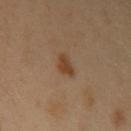Captured during whole-body skin photography for melanoma surveillance; the lesion was not biopsied.
A male patient, aged 38 to 42.
Captured under cross-polarized illumination.
On the left upper arm.
Measured at roughly 2.5 mm in maximum diameter.
A 15 mm crop from a total-body photograph taken for skin-cancer surveillance.
The lesion-visualizer software estimated a lesion area of about 3.5 mm², an outline eccentricity of about 0.75 (0 = round, 1 = elongated), and two-axis asymmetry of about 0.35. And it measured internal color variation of about 1 on a 0–10 scale and peripheral color asymmetry of about 0.5.